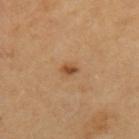Imaged during a routine full-body skin examination; the lesion was not biopsied and no histopathology is available.
A male patient roughly 65 years of age.
From the left upper arm.
The recorded lesion diameter is about 2 mm.
Captured under cross-polarized illumination.
Cropped from a whole-body photographic skin survey; the tile spans about 15 mm.